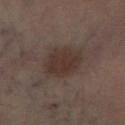{"biopsy_status": "not biopsied; imaged during a skin examination", "lesion_size": {"long_diameter_mm_approx": 4.0}, "automated_metrics": {"border_irregularity_0_10": 2.0, "color_variation_0_10": 2.5, "peripheral_color_asymmetry": 1.0, "lesion_detection_confidence_0_100": 100}, "image": {"source": "total-body photography crop", "field_of_view_mm": 15}, "site": "right lower leg"}